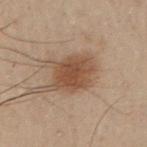Q: Is there a histopathology result?
A: no biopsy performed (imaged during a skin exam)
Q: What is the anatomic site?
A: the left arm
Q: What are the patient's age and sex?
A: male, in their 50s
Q: Lesion size?
A: about 4.5 mm
Q: How was the tile lit?
A: cross-polarized illumination
Q: What is the imaging modality?
A: ~15 mm tile from a whole-body skin photo